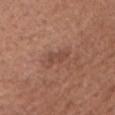Q: Is there a histopathology result?
A: catalogued during a skin exam; not biopsied
Q: Who is the patient?
A: male, aged 63–67
Q: How was this image acquired?
A: ~15 mm crop, total-body skin-cancer survey
Q: What is the anatomic site?
A: the head or neck